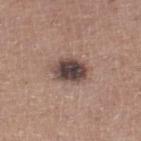<tbp_lesion>
  <biopsy_status>not biopsied; imaged during a skin examination</biopsy_status>
  <image>
    <source>total-body photography crop</source>
    <field_of_view_mm>15</field_of_view_mm>
  </image>
  <site>right lower leg</site>
  <lighting>white-light</lighting>
  <patient>
    <sex>male</sex>
    <age_approx>60</age_approx>
  </patient>
</tbp_lesion>